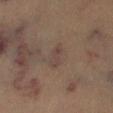The lesion was photographed on a routine skin check and not biopsied; there is no pathology result.
A roughly 15 mm field-of-view crop from a total-body skin photograph.
A male subject in their mid- to late 40s.
The lesion is located on the left lower leg.
An algorithmic analysis of the crop reported an outline eccentricity of about 0.85 (0 = round, 1 = elongated) and a shape-asymmetry score of about 0.35 (0 = symmetric). The software also gave border irregularity of about 3.5 on a 0–10 scale, a color-variation rating of about 1.5/10, and peripheral color asymmetry of about 0.5.